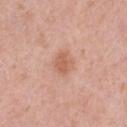Case summary:
– workup · imaged on a skin check; not biopsied
– illumination · white-light
– subject · female, in their 40s
– acquisition · 15 mm crop, total-body photography
– site · the left thigh
– lesion diameter · about 2.5 mm